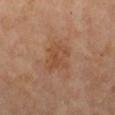workup = imaged on a skin check; not biopsied
TBP lesion metrics = a mean CIELAB color near L≈48 a*≈21 b*≈33, a lesion–skin lightness drop of about 7, and a normalized border contrast of about 6; a border-irregularity rating of about 4/10, a within-lesion color-variation index near 3/10, and a peripheral color-asymmetry measure near 1
location = the chest
patient = female, about 60 years old
acquisition = total-body-photography crop, ~15 mm field of view
lighting = cross-polarized